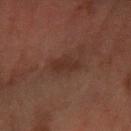* follow-up — catalogued during a skin exam; not biopsied
* subject — male, aged 58–62
* illumination — cross-polarized illumination
* TBP lesion metrics — a lesion area of about 5 mm², a shape eccentricity near 0.85, and two-axis asymmetry of about 0.25; a within-lesion color-variation index near 2/10; a classifier nevus-likeness of about 5/100 and lesion-presence confidence of about 100/100
* image source — ~15 mm tile from a whole-body skin photo
* anatomic site — the left forearm